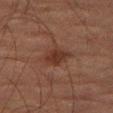Clinical impression:
Part of a total-body skin-imaging series; this lesion was reviewed on a skin check and was not flagged for biopsy.
Image and clinical context:
A male subject in their mid- to late 70s. An algorithmic analysis of the crop reported a lesion color around L≈27 a*≈17 b*≈22 in CIELAB, about 6 CIELAB-L* units darker than the surrounding skin, and a normalized lesion–skin contrast near 7. The analysis additionally found an automated nevus-likeness rating near 40 out of 100. Captured under cross-polarized illumination. A roughly 15 mm field-of-view crop from a total-body skin photograph. The lesion is on the right thigh. Approximately 3.5 mm at its widest.